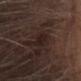{"biopsy_status": "not biopsied; imaged during a skin examination", "image": {"source": "total-body photography crop", "field_of_view_mm": 15}, "patient": {"sex": "male", "age_approx": 70}, "site": "head or neck"}